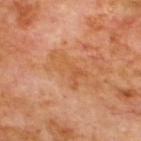Q: Was this lesion biopsied?
A: catalogued during a skin exam; not biopsied
Q: What is the anatomic site?
A: the back
Q: Patient demographics?
A: male, aged around 70
Q: What lighting was used for the tile?
A: cross-polarized
Q: What kind of image is this?
A: total-body-photography crop, ~15 mm field of view